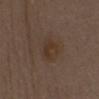Q: Was a biopsy performed?
A: no biopsy performed (imaged during a skin exam)
Q: What is the anatomic site?
A: the front of the torso
Q: Who is the patient?
A: male, aged around 70
Q: What is the imaging modality?
A: total-body-photography crop, ~15 mm field of view
Q: What lighting was used for the tile?
A: white-light